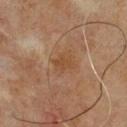A 15 mm close-up extracted from a 3D total-body photography capture. A male patient about 75 years old. The lesion is located on the front of the torso. Approximately 2.5 mm at its widest.